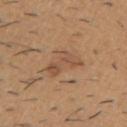Findings:
– notes — no biopsy performed (imaged during a skin exam)
– acquisition — total-body-photography crop, ~15 mm field of view
– tile lighting — white-light illumination
– lesion size — about 5 mm
– subject — male, approximately 60 years of age
– anatomic site — the upper back